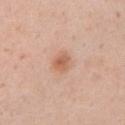biopsy status: catalogued during a skin exam; not biopsied | image-analysis metrics: a footprint of about 4 mm², an eccentricity of roughly 0.55, and a shape-asymmetry score of about 0.2 (0 = symmetric); a mean CIELAB color near L≈61 a*≈23 b*≈33, a lesion–skin lightness drop of about 10, and a lesion-to-skin contrast of about 7 (normalized; higher = more distinct) | lesion size: ~2.5 mm (longest diameter) | subject: female, aged around 35 | site: the left upper arm | tile lighting: white-light illumination | image: total-body-photography crop, ~15 mm field of view.